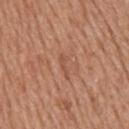workup = imaged on a skin check; not biopsied | anatomic site = the upper back | diameter = about 2.5 mm | imaging modality = 15 mm crop, total-body photography | patient = male, roughly 60 years of age | tile lighting = white-light.